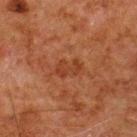Q: Is there a histopathology result?
A: total-body-photography surveillance lesion; no biopsy
Q: Who is the patient?
A: male, approximately 80 years of age
Q: What lighting was used for the tile?
A: cross-polarized illumination
Q: Lesion location?
A: the upper back
Q: How was this image acquired?
A: total-body-photography crop, ~15 mm field of view
Q: Lesion size?
A: about 3 mm